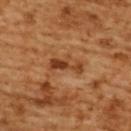Q: Where on the body is the lesion?
A: the upper back
Q: Automated lesion metrics?
A: a border-irregularity index near 6.5/10, a within-lesion color-variation index near 2/10, and peripheral color asymmetry of about 0
Q: How was the tile lit?
A: cross-polarized illumination
Q: Lesion size?
A: ~4 mm (longest diameter)
Q: Patient demographics?
A: female, approximately 55 years of age
Q: How was this image acquired?
A: ~15 mm crop, total-body skin-cancer survey